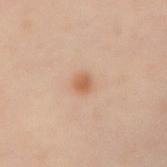biopsy status=no biopsy performed (imaged during a skin exam)
acquisition=15 mm crop, total-body photography
subject=female, roughly 40 years of age
size=≈2 mm
image-analysis metrics=a footprint of about 3 mm², an eccentricity of roughly 0.4, and two-axis asymmetry of about 0.25; a detector confidence of about 100 out of 100 that the crop contains a lesion
tile lighting=cross-polarized
anatomic site=the right forearm